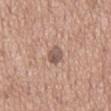Captured during whole-body skin photography for melanoma surveillance; the lesion was not biopsied. Imaged with white-light lighting. A lesion tile, about 15 mm wide, cut from a 3D total-body photograph. The recorded lesion diameter is about 3 mm. The total-body-photography lesion software estimated an average lesion color of about L≈54 a*≈17 b*≈24 (CIELAB), a lesion–skin lightness drop of about 11, and a normalized border contrast of about 8. It also reported a border-irregularity index near 2/10, a within-lesion color-variation index near 4/10, and radial color variation of about 2. A male patient roughly 70 years of age. On the front of the torso.